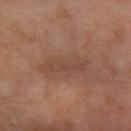Impression:
Recorded during total-body skin imaging; not selected for excision or biopsy.
Clinical summary:
Automated tile analysis of the lesion measured an area of roughly 10 mm², an outline eccentricity of about 0.95 (0 = round, 1 = elongated), and two-axis asymmetry of about 0.25. It also reported a nevus-likeness score of about 0/100. A region of skin cropped from a whole-body photographic capture, roughly 15 mm wide. A male patient, about 60 years old. The lesion is located on the arm.